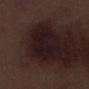<tbp_lesion>
  <biopsy_status>not biopsied; imaged during a skin examination</biopsy_status>
  <image>
    <source>total-body photography crop</source>
    <field_of_view_mm>15</field_of_view_mm>
  </image>
  <site>left lower leg</site>
  <automated_metrics>
    <shape_asymmetry>0.3</shape_asymmetry>
    <border_irregularity_0_10>4.0</border_irregularity_0_10>
    <color_variation_0_10>2.0</color_variation_0_10>
    <peripheral_color_asymmetry>0.5</peripheral_color_asymmetry>
    <lesion_detection_confidence_0_100>75</lesion_detection_confidence_0_100>
  </automated_metrics>
  <patient>
    <sex>male</sex>
    <age_approx>70</age_approx>
  </patient>
  <lesion_size>
    <long_diameter_mm_approx>4.5</long_diameter_mm_approx>
  </lesion_size>
  <lighting>white-light</lighting>
</tbp_lesion>